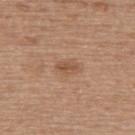Q: Was this lesion biopsied?
A: imaged on a skin check; not biopsied
Q: What kind of image is this?
A: ~15 mm tile from a whole-body skin photo
Q: Lesion size?
A: ≈2.5 mm
Q: What lighting was used for the tile?
A: white-light illumination
Q: Lesion location?
A: the upper back
Q: Automated lesion metrics?
A: a mean CIELAB color near L≈52 a*≈20 b*≈32, a lesion–skin lightness drop of about 8, and a normalized border contrast of about 6.5; a color-variation rating of about 1.5/10
Q: What are the patient's age and sex?
A: female, aged around 65